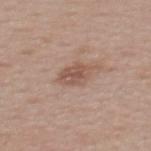biopsy status = no biopsy performed (imaged during a skin exam) | site = the upper back | size = about 3 mm | illumination = white-light | patient = female, about 55 years old | image source = ~15 mm tile from a whole-body skin photo | automated metrics = a normalized border contrast of about 6.5; a border-irregularity index near 2/10, a within-lesion color-variation index near 3.5/10, and radial color variation of about 1; a classifier nevus-likeness of about 5/100 and lesion-presence confidence of about 100/100.